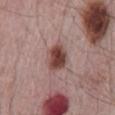Clinical impression: This lesion was catalogued during total-body skin photography and was not selected for biopsy. Context: About 4 mm across. This image is a 15 mm lesion crop taken from a total-body photograph. Captured under white-light illumination. The lesion is on the mid back. The subject is a male roughly 65 years of age.